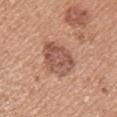Impression: The lesion was tiled from a total-body skin photograph and was not biopsied. Clinical summary: Measured at roughly 4.5 mm in maximum diameter. An algorithmic analysis of the crop reported a footprint of about 13 mm², an outline eccentricity of about 0.6 (0 = round, 1 = elongated), and a shape-asymmetry score of about 0.15 (0 = symmetric). And it measured a lesion–skin lightness drop of about 11 and a normalized lesion–skin contrast near 7.5. Cropped from a total-body skin-imaging series; the visible field is about 15 mm. The tile uses white-light illumination. The lesion is located on the left upper arm. A female subject aged 33–37.